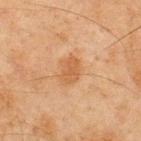Impression:
Imaged during a routine full-body skin examination; the lesion was not biopsied and no histopathology is available.
Acquisition and patient details:
A 15 mm crop from a total-body photograph taken for skin-cancer surveillance. A male patient aged 43 to 47. Imaged with cross-polarized lighting. The lesion is located on the upper back.